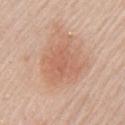Q: Was a biopsy performed?
A: total-body-photography surveillance lesion; no biopsy